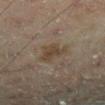Q: Is there a histopathology result?
A: no biopsy performed (imaged during a skin exam)
Q: Who is the patient?
A: male, aged approximately 45
Q: What lighting was used for the tile?
A: cross-polarized
Q: Automated lesion metrics?
A: an area of roughly 6.5 mm², an outline eccentricity of about 0.4 (0 = round, 1 = elongated), and a symmetry-axis asymmetry near 0.45
Q: What is the anatomic site?
A: the left lower leg
Q: How was this image acquired?
A: ~15 mm crop, total-body skin-cancer survey
Q: What is the lesion's diameter?
A: about 3 mm